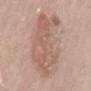Captured during whole-body skin photography for melanoma surveillance; the lesion was not biopsied. A female patient aged 63–67. A 15 mm crop from a total-body photograph taken for skin-cancer surveillance. Located on the mid back. This is a white-light tile. Measured at roughly 10 mm in maximum diameter. The total-body-photography lesion software estimated an average lesion color of about L≈60 a*≈17 b*≈26 (CIELAB), roughly 8 lightness units darker than nearby skin, and a normalized lesion–skin contrast near 5.5. And it measured peripheral color asymmetry of about 1.5.